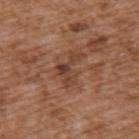biopsy_status: not biopsied; imaged during a skin examination
patient:
  sex: male
  age_approx: 75
site: upper back
automated_metrics:
  cielab_L: 42
  cielab_a: 21
  cielab_b: 29
  vs_skin_darker_L: 8.0
  vs_skin_contrast_norm: 7.0
image:
  source: total-body photography crop
  field_of_view_mm: 15